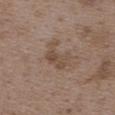| key | value |
|---|---|
| workup | no biopsy performed (imaged during a skin exam) |
| anatomic site | the upper back |
| subject | female, roughly 35 years of age |
| acquisition | ~15 mm tile from a whole-body skin photo |